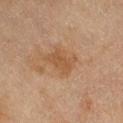Clinical impression: Imaged during a routine full-body skin examination; the lesion was not biopsied and no histopathology is available. Context: This is a cross-polarized tile. Automated tile analysis of the lesion measured a footprint of about 8 mm², an eccentricity of roughly 0.7, and two-axis asymmetry of about 0.3. Cropped from a whole-body photographic skin survey; the tile spans about 15 mm. The patient is a female roughly 60 years of age. The lesion is located on the right thigh. Approximately 4 mm at its widest.